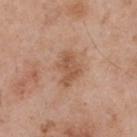A male subject aged 53–57.
On the upper back.
A roughly 15 mm field-of-view crop from a total-body skin photograph.
Captured under white-light illumination.
Approximately 4 mm at its widest.
Automated tile analysis of the lesion measured a lesion area of about 8 mm² and an eccentricity of roughly 0.75. The software also gave an average lesion color of about L≈55 a*≈21 b*≈32 (CIELAB), about 9 CIELAB-L* units darker than the surrounding skin, and a normalized border contrast of about 6.5.